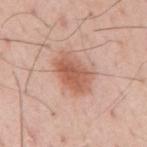biopsy status: no biopsy performed (imaged during a skin exam) | site: the back | lighting: white-light | subject: male, roughly 55 years of age | image: ~15 mm crop, total-body skin-cancer survey | automated lesion analysis: an outline eccentricity of about 0.8 (0 = round, 1 = elongated) and a symmetry-axis asymmetry near 0.15; a mean CIELAB color near L≈59 a*≈24 b*≈30 and roughly 11 lightness units darker than nearby skin; a border-irregularity index near 2.5/10, a color-variation rating of about 4.5/10, and a peripheral color-asymmetry measure near 1.5 | lesion diameter: about 5 mm.